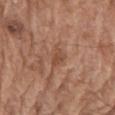Assessment:
Imaged during a routine full-body skin examination; the lesion was not biopsied and no histopathology is available.
Clinical summary:
This is a white-light tile. A region of skin cropped from a whole-body photographic capture, roughly 15 mm wide. The lesion is on the chest. A male subject in their mid-70s.